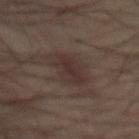Findings:
– follow-up · imaged on a skin check; not biopsied
– size · ≈5 mm
– lighting · cross-polarized
– image · ~15 mm crop, total-body skin-cancer survey
– location · the abdomen
– patient · male, aged 48–52